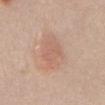notes: catalogued during a skin exam; not biopsied | automated metrics: a footprint of about 14 mm² and an eccentricity of roughly 0.8; a lesion–skin lightness drop of about 7 and a lesion-to-skin contrast of about 4.5 (normalized; higher = more distinct); a nevus-likeness score of about 85/100 and lesion-presence confidence of about 100/100 | patient: female, approximately 55 years of age | image source: 15 mm crop, total-body photography | tile lighting: white-light illumination | body site: the abdomen | lesion diameter: about 5.5 mm.